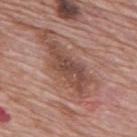<case>
  <biopsy_status>not biopsied; imaged during a skin examination</biopsy_status>
  <site>mid back</site>
  <patient>
    <sex>male</sex>
    <age_approx>70</age_approx>
  </patient>
  <image>
    <source>total-body photography crop</source>
    <field_of_view_mm>15</field_of_view_mm>
  </image>
</case>